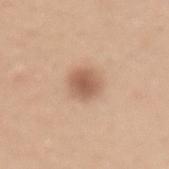follow-up — catalogued during a skin exam; not biopsied
location — the lower back
subject — male, in their 50s
tile lighting — white-light illumination
image — ~15 mm crop, total-body skin-cancer survey
image-analysis metrics — about 12 CIELAB-L* units darker than the surrounding skin and a normalized lesion–skin contrast near 8
lesion size — ≈3.5 mm